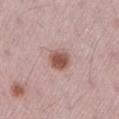Impression:
Imaged during a routine full-body skin examination; the lesion was not biopsied and no histopathology is available.
Acquisition and patient details:
Imaged with white-light lighting. A 15 mm crop from a total-body photograph taken for skin-cancer surveillance. A female subject, aged around 45. The lesion is on the right upper arm.